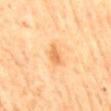Q: Is there a histopathology result?
A: no biopsy performed (imaged during a skin exam)
Q: What is the anatomic site?
A: the back
Q: Illumination type?
A: cross-polarized illumination
Q: Lesion size?
A: ~2.5 mm (longest diameter)
Q: What are the patient's age and sex?
A: female, in their mid- to late 60s
Q: What kind of image is this?
A: ~15 mm tile from a whole-body skin photo